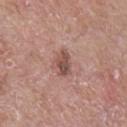This lesion was catalogued during total-body skin photography and was not selected for biopsy. Captured under white-light illumination. A male patient, in their mid-60s. Measured at roughly 3 mm in maximum diameter. A 15 mm close-up extracted from a 3D total-body photography capture. An algorithmic analysis of the crop reported an eccentricity of roughly 0.8. The software also gave border irregularity of about 2.5 on a 0–10 scale and a color-variation rating of about 5.5/10. It also reported an automated nevus-likeness rating near 30 out of 100. The lesion is on the chest.